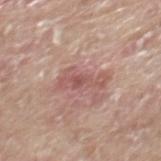Clinical impression: The lesion was tiled from a total-body skin photograph and was not biopsied. Clinical summary: A 15 mm crop from a total-body photograph taken for skin-cancer surveillance. A male patient, aged approximately 65. About 4.5 mm across. From the back.